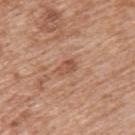No biopsy was performed on this lesion — it was imaged during a full skin examination and was not determined to be concerning. Captured under white-light illumination. Approximately 2.5 mm at its widest. Cropped from a whole-body photographic skin survey; the tile spans about 15 mm. The patient is a male roughly 60 years of age. The lesion is on the back. An algorithmic analysis of the crop reported an outline eccentricity of about 0.75 (0 = round, 1 = elongated) and a symmetry-axis asymmetry near 0.3.